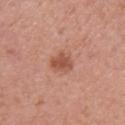Q: Was this lesion biopsied?
A: imaged on a skin check; not biopsied
Q: What are the patient's age and sex?
A: female, roughly 50 years of age
Q: Lesion size?
A: ~3 mm (longest diameter)
Q: Automated lesion metrics?
A: an average lesion color of about L≈53 a*≈26 b*≈31 (CIELAB), a lesion–skin lightness drop of about 10, and a normalized lesion–skin contrast near 7.5; an automated nevus-likeness rating near 80 out of 100 and lesion-presence confidence of about 100/100
Q: Lesion location?
A: the right upper arm
Q: What is the imaging modality?
A: total-body-photography crop, ~15 mm field of view
Q: What lighting was used for the tile?
A: white-light illumination